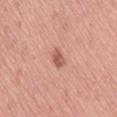follow-up: catalogued during a skin exam; not biopsied | lesion size: ~3 mm (longest diameter) | subject: female, approximately 55 years of age | tile lighting: white-light | image source: total-body-photography crop, ~15 mm field of view | location: the right thigh.